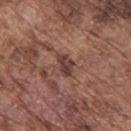The lesion was photographed on a routine skin check and not biopsied; there is no pathology result. A 15 mm close-up tile from a total-body photography series done for melanoma screening. The subject is a male in their mid-70s. Located on the upper back. Approximately 2.5 mm at its widest. This is a white-light tile.